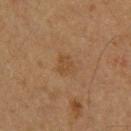{
  "biopsy_status": "not biopsied; imaged during a skin examination",
  "image": {
    "source": "total-body photography crop",
    "field_of_view_mm": 15
  },
  "lighting": "cross-polarized",
  "lesion_size": {
    "long_diameter_mm_approx": 2.5
  },
  "patient": {
    "sex": "male",
    "age_approx": 60
  }
}